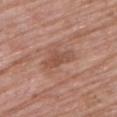Findings:
– diameter: about 4 mm
– illumination: white-light
– automated lesion analysis: an area of roughly 7 mm², a shape eccentricity near 0.85, and a shape-asymmetry score of about 0.4 (0 = symmetric)
– image source: total-body-photography crop, ~15 mm field of view
– location: the back
– patient: male, aged 83 or over